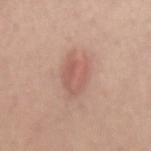Cropped from a whole-body photographic skin survey; the tile spans about 15 mm. Longest diameter approximately 5 mm. This is a white-light tile. Located on the lower back. A male patient about 30 years old.